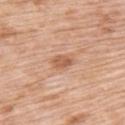A 15 mm crop from a total-body photograph taken for skin-cancer surveillance. Measured at roughly 3 mm in maximum diameter. The tile uses white-light illumination. A female subject aged approximately 70. The lesion is located on the left upper arm.